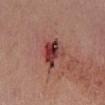Findings:
- notes · catalogued during a skin exam; not biopsied
- lighting · white-light
- lesion diameter · ~3.5 mm (longest diameter)
- location · the left lower leg
- image-analysis metrics · lesion-presence confidence of about 100/100
- patient · female, in their 40s
- imaging modality · ~15 mm crop, total-body skin-cancer survey